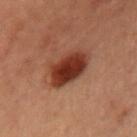biopsy_status: not biopsied; imaged during a skin examination
image:
  source: total-body photography crop
  field_of_view_mm: 15
lesion_size:
  long_diameter_mm_approx: 5.5
lighting: cross-polarized
patient:
  sex: female
  age_approx: 55
automated_metrics:
  border_irregularity_0_10: 2.0
  color_variation_0_10: 4.0
  nevus_likeness_0_100: 100
  lesion_detection_confidence_0_100: 100
site: chest